Imaged with cross-polarized lighting; a male patient, aged 43 to 47; the lesion is located on the mid back; the lesion's longest dimension is about 6 mm; cropped from a whole-body photographic skin survey; the tile spans about 15 mm.
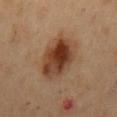Q: What did pathology find?
A: an atypical melanocytic neoplasm — a lesion of indeterminate malignant potential